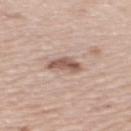Notes:
- biopsy status: imaged on a skin check; not biopsied
- lighting: white-light illumination
- image-analysis metrics: a footprint of about 6 mm², an eccentricity of roughly 0.8, and two-axis asymmetry of about 0.3; a border-irregularity index near 3.5/10, a within-lesion color-variation index near 4/10, and a peripheral color-asymmetry measure near 1.5
- anatomic site: the arm
- patient: female, about 50 years old
- acquisition: total-body-photography crop, ~15 mm field of view
- lesion size: about 3.5 mm